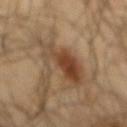Notes:
– workup · imaged on a skin check; not biopsied
– image · total-body-photography crop, ~15 mm field of view
– TBP lesion metrics · an outline eccentricity of about 0.9 (0 = round, 1 = elongated); a lesion color around L≈42 a*≈16 b*≈29 in CIELAB and about 10 CIELAB-L* units darker than the surrounding skin; a lesion-detection confidence of about 100/100
– patient · male, roughly 65 years of age
– body site · the mid back